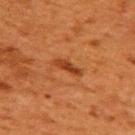Assessment: The lesion was tiled from a total-body skin photograph and was not biopsied. Acquisition and patient details: The lesion is on the upper back. A 15 mm close-up tile from a total-body photography series done for melanoma screening. Captured under cross-polarized illumination. A female subject, roughly 50 years of age. About 3.5 mm across.